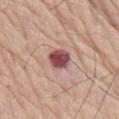This lesion was catalogued during total-body skin photography and was not selected for biopsy. Cropped from a total-body skin-imaging series; the visible field is about 15 mm. Captured under white-light illumination. The patient is a male roughly 80 years of age. The lesion is located on the chest. Automated tile analysis of the lesion measured a lesion color around L≈49 a*≈27 b*≈19 in CIELAB, roughly 18 lightness units darker than nearby skin, and a lesion-to-skin contrast of about 12.5 (normalized; higher = more distinct). The analysis additionally found border irregularity of about 1.5 on a 0–10 scale and internal color variation of about 5 on a 0–10 scale. The analysis additionally found a nevus-likeness score of about 20/100.